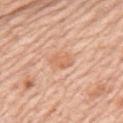notes = imaged on a skin check; not biopsied
subject = male, roughly 80 years of age
imaging modality = 15 mm crop, total-body photography
location = the left upper arm